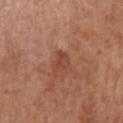Imaged during a routine full-body skin examination; the lesion was not biopsied and no histopathology is available.